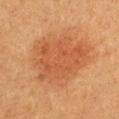A female patient, in their 30s. This is a cross-polarized tile. Longest diameter approximately 7.5 mm. A lesion tile, about 15 mm wide, cut from a 3D total-body photograph. The lesion is on the chest.